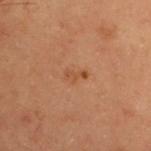workup = no biopsy performed (imaged during a skin exam); patient = female, aged around 40; location = the upper back; imaging modality = ~15 mm tile from a whole-body skin photo.